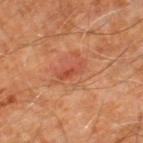| feature | finding |
|---|---|
| workup | imaged on a skin check; not biopsied |
| site | the right leg |
| subject | male, about 60 years old |
| imaging modality | ~15 mm tile from a whole-body skin photo |
| lighting | cross-polarized illumination |